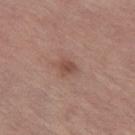Case summary:
• biopsy status · imaged on a skin check; not biopsied
• location · the left thigh
• acquisition · ~15 mm tile from a whole-body skin photo
• automated metrics · a footprint of about 3.5 mm², an outline eccentricity of about 0.6 (0 = round, 1 = elongated), and a symmetry-axis asymmetry near 0.25; about 9 CIELAB-L* units darker than the surrounding skin; a border-irregularity index near 2.5/10; a nevus-likeness score of about 15/100 and lesion-presence confidence of about 100/100
• patient · female, roughly 65 years of age